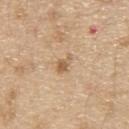{"biopsy_status": "not biopsied; imaged during a skin examination", "site": "upper back", "patient": {"sex": "male", "age_approx": 70}, "lighting": "white-light", "image": {"source": "total-body photography crop", "field_of_view_mm": 15}, "lesion_size": {"long_diameter_mm_approx": 2.5}}